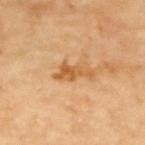Q: Was this lesion biopsied?
A: imaged on a skin check; not biopsied
Q: What lighting was used for the tile?
A: cross-polarized
Q: What is the anatomic site?
A: the upper back
Q: What kind of image is this?
A: ~15 mm crop, total-body skin-cancer survey
Q: What is the lesion's diameter?
A: ≈4 mm
Q: Automated lesion metrics?
A: a lesion area of about 5.5 mm² and a shape-asymmetry score of about 0.4 (0 = symmetric); a lesion–skin lightness drop of about 11 and a normalized lesion–skin contrast near 7.5; border irregularity of about 5.5 on a 0–10 scale and internal color variation of about 2.5 on a 0–10 scale
Q: Who is the patient?
A: female, aged 63–67